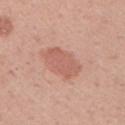This lesion was catalogued during total-body skin photography and was not selected for biopsy.
A male subject approximately 45 years of age.
A 15 mm close-up extracted from a 3D total-body photography capture.
The lesion is on the left upper arm.
Longest diameter approximately 4.5 mm.